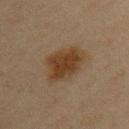<lesion>
  <automated_metrics>
    <area_mm2_approx>15.0</area_mm2_approx>
    <eccentricity>0.7</eccentricity>
    <shape_asymmetry>0.2</shape_asymmetry>
    <vs_skin_darker_L>9.0</vs_skin_darker_L>
    <vs_skin_contrast_norm>9.5</vs_skin_contrast_norm>
    <border_irregularity_0_10>2.5</border_irregularity_0_10>
    <color_variation_0_10>3.0</color_variation_0_10>
    <peripheral_color_asymmetry>1.0</peripheral_color_asymmetry>
  </automated_metrics>
  <patient>
    <sex>female</sex>
    <age_approx>45</age_approx>
  </patient>
  <lesion_size>
    <long_diameter_mm_approx>5.0</long_diameter_mm_approx>
  </lesion_size>
  <site>left upper arm</site>
  <image>
    <source>total-body photography crop</source>
    <field_of_view_mm>15</field_of_view_mm>
  </image>
  <lighting>cross-polarized</lighting>
</lesion>